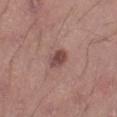| field | value |
|---|---|
| notes | no biopsy performed (imaged during a skin exam) |
| automated metrics | a footprint of about 4 mm² and a symmetry-axis asymmetry near 0.15; an average lesion color of about L≈46 a*≈21 b*≈22 (CIELAB), about 12 CIELAB-L* units darker than the surrounding skin, and a normalized border contrast of about 9; a border-irregularity index near 1.5/10; a classifier nevus-likeness of about 55/100 and a detector confidence of about 100 out of 100 that the crop contains a lesion |
| subject | male, in their mid-40s |
| image | ~15 mm tile from a whole-body skin photo |
| lesion size | ≈2.5 mm |
| tile lighting | white-light |
| site | the right thigh |